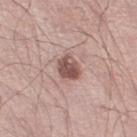Case summary:
– workup: total-body-photography surveillance lesion; no biopsy
– subject: male, about 55 years old
– image: total-body-photography crop, ~15 mm field of view
– site: the leg